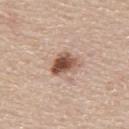Clinical impression:
No biopsy was performed on this lesion — it was imaged during a full skin examination and was not determined to be concerning.
Context:
From the upper back. A lesion tile, about 15 mm wide, cut from a 3D total-body photograph. A male patient, roughly 60 years of age.